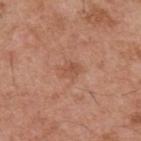Recorded during total-body skin imaging; not selected for excision or biopsy.
Located on the upper back.
Automated tile analysis of the lesion measured a nevus-likeness score of about 0/100.
A 15 mm crop from a total-body photograph taken for skin-cancer surveillance.
Imaged with white-light lighting.
Measured at roughly 3 mm in maximum diameter.
The patient is a male about 55 years old.